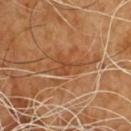Context:
On the chest. A 15 mm close-up tile from a total-body photography series done for melanoma screening. Imaged with cross-polarized lighting. Approximately 3.5 mm at its widest. A male patient, aged 63 to 67.